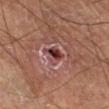This lesion was catalogued during total-body skin photography and was not selected for biopsy. A male patient roughly 55 years of age. Captured under cross-polarized illumination. Cropped from a total-body skin-imaging series; the visible field is about 15 mm. The lesion's longest dimension is about 3 mm. The lesion is located on the right lower leg.